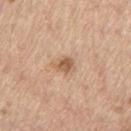This lesion was catalogued during total-body skin photography and was not selected for biopsy.
About 3 mm across.
Captured under white-light illumination.
This image is a 15 mm lesion crop taken from a total-body photograph.
The lesion is on the left thigh.
A male subject roughly 70 years of age.